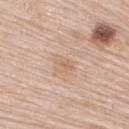Q: Was this lesion biopsied?
A: catalogued during a skin exam; not biopsied
Q: What is the anatomic site?
A: the right upper arm
Q: How was the tile lit?
A: white-light illumination
Q: How was this image acquired?
A: ~15 mm crop, total-body skin-cancer survey
Q: What are the patient's age and sex?
A: female, approximately 65 years of age
Q: How large is the lesion?
A: ~2.5 mm (longest diameter)